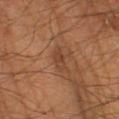{
  "biopsy_status": "not biopsied; imaged during a skin examination"
}